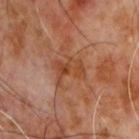This lesion was catalogued during total-body skin photography and was not selected for biopsy. From the chest. A male subject aged 58–62. Imaged with cross-polarized lighting. The lesion's longest dimension is about 3.5 mm. A roughly 15 mm field-of-view crop from a total-body skin photograph.